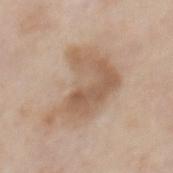workup = imaged on a skin check; not biopsied | image = ~15 mm tile from a whole-body skin photo | site = the mid back | subject = male, aged approximately 85 or older.